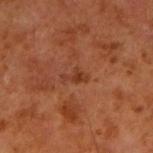Q: Is there a histopathology result?
A: total-body-photography surveillance lesion; no biopsy
Q: What are the patient's age and sex?
A: male, aged 58–62
Q: Illumination type?
A: cross-polarized illumination
Q: Lesion size?
A: ≈3 mm
Q: Where on the body is the lesion?
A: the right upper arm
Q: What is the imaging modality?
A: 15 mm crop, total-body photography
Q: What did automated image analysis measure?
A: a footprint of about 2.5 mm², an eccentricity of roughly 0.85, and a symmetry-axis asymmetry near 0.5; a border-irregularity rating of about 5.5/10, a within-lesion color-variation index near 0/10, and radial color variation of about 0